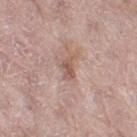The lesion was photographed on a routine skin check and not biopsied; there is no pathology result.
The lesion's longest dimension is about 3 mm.
The lesion is on the right thigh.
Cropped from a whole-body photographic skin survey; the tile spans about 15 mm.
A female subject, approximately 70 years of age.
Automated tile analysis of the lesion measured a lesion area of about 3.5 mm², an eccentricity of roughly 0.85, and a shape-asymmetry score of about 0.4 (0 = symmetric). And it measured a border-irregularity index near 4/10, internal color variation of about 2 on a 0–10 scale, and a peripheral color-asymmetry measure near 0.5. The analysis additionally found a nevus-likeness score of about 0/100 and a detector confidence of about 100 out of 100 that the crop contains a lesion.
Imaged with white-light lighting.